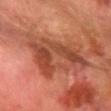Recorded during total-body skin imaging; not selected for excision or biopsy. The tile uses cross-polarized illumination. From the left forearm. Approximately 7.5 mm at its widest. A roughly 15 mm field-of-view crop from a total-body skin photograph. The patient is a male roughly 80 years of age. Automated image analysis of the tile measured an area of roughly 19 mm², an outline eccentricity of about 0.85 (0 = round, 1 = elongated), and a shape-asymmetry score of about 0.65 (0 = symmetric). And it measured a lesion color around L≈34 a*≈23 b*≈27 in CIELAB, a lesion–skin lightness drop of about 8, and a normalized border contrast of about 7. The analysis additionally found a color-variation rating of about 5/10 and a peripheral color-asymmetry measure near 2. It also reported an automated nevus-likeness rating near 0 out of 100 and lesion-presence confidence of about 100/100.